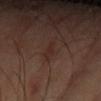Assessment: The lesion was photographed on a routine skin check and not biopsied; there is no pathology result. Context: On the left forearm. A 15 mm close-up extracted from a 3D total-body photography capture. Captured under cross-polarized illumination. Measured at roughly 2.5 mm in maximum diameter. The lesion-visualizer software estimated an average lesion color of about L≈27 a*≈17 b*≈22 (CIELAB) and a normalized border contrast of about 5.5. And it measured a border-irregularity index near 4.5/10, internal color variation of about 0 on a 0–10 scale, and radial color variation of about 0. The analysis additionally found a detector confidence of about 95 out of 100 that the crop contains a lesion. The subject is a female approximately 55 years of age.